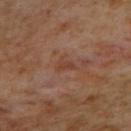Clinical impression: No biopsy was performed on this lesion — it was imaged during a full skin examination and was not determined to be concerning. Image and clinical context: The tile uses cross-polarized illumination. The lesion is on the upper back. The lesion-visualizer software estimated an eccentricity of roughly 0.75 and a symmetry-axis asymmetry near 0.5. It also reported a lesion color around L≈40 a*≈21 b*≈28 in CIELAB, a lesion–skin lightness drop of about 6, and a lesion-to-skin contrast of about 5.5 (normalized; higher = more distinct). It also reported a border-irregularity index near 4.5/10, internal color variation of about 1 on a 0–10 scale, and peripheral color asymmetry of about 0.5. The analysis additionally found an automated nevus-likeness rating near 0 out of 100 and lesion-presence confidence of about 100/100. A female patient in their mid-60s. Longest diameter approximately 2.5 mm. A region of skin cropped from a whole-body photographic capture, roughly 15 mm wide.